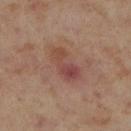Clinical impression: This lesion was catalogued during total-body skin photography and was not selected for biopsy. Context: Measured at roughly 4.5 mm in maximum diameter. A 15 mm crop from a total-body photograph taken for skin-cancer surveillance. The total-body-photography lesion software estimated an average lesion color of about L≈46 a*≈23 b*≈25 (CIELAB) and a normalized border contrast of about 6.5. The software also gave a classifier nevus-likeness of about 0/100 and a detector confidence of about 100 out of 100 that the crop contains a lesion. The tile uses cross-polarized illumination. The patient is a female roughly 55 years of age. Located on the right lower leg.